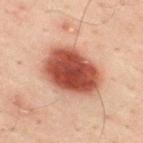notes = imaged on a skin check; not biopsied.